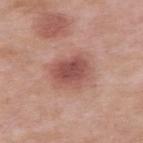This lesion was catalogued during total-body skin photography and was not selected for biopsy.
On the left upper arm.
Measured at roughly 4 mm in maximum diameter.
The tile uses white-light illumination.
A male subject, roughly 55 years of age.
This image is a 15 mm lesion crop taken from a total-body photograph.